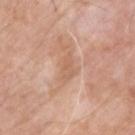{
  "biopsy_status": "not biopsied; imaged during a skin examination",
  "site": "upper back",
  "patient": {
    "sex": "male",
    "age_approx": 65
  },
  "lighting": "white-light",
  "automated_metrics": {
    "area_mm2_approx": 4.0,
    "eccentricity": 0.8,
    "shape_asymmetry": 0.3,
    "nevus_likeness_0_100": 0,
    "lesion_detection_confidence_0_100": 100
  },
  "image": {
    "source": "total-body photography crop",
    "field_of_view_mm": 15
  }
}